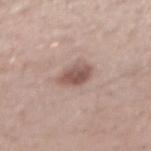No biopsy was performed on this lesion — it was imaged during a full skin examination and was not determined to be concerning.
This is a white-light tile.
The lesion is on the right forearm.
The patient is a male about 40 years old.
A 15 mm close-up tile from a total-body photography series done for melanoma screening.
The lesion-visualizer software estimated peripheral color asymmetry of about 1.
Longest diameter approximately 3.5 mm.